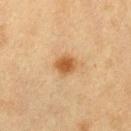follow-up — imaged on a skin check; not biopsied
lesion diameter — about 3 mm
patient — female, about 40 years old
body site — the left thigh
image — 15 mm crop, total-body photography
tile lighting — cross-polarized